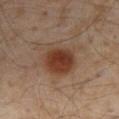{"biopsy_status": "not biopsied; imaged during a skin examination", "lesion_size": {"long_diameter_mm_approx": 5.0}, "site": "abdomen", "patient": {"sex": "male", "age_approx": 60}, "image": {"source": "total-body photography crop", "field_of_view_mm": 15}, "automated_metrics": {"area_mm2_approx": 16.0, "eccentricity": 0.45, "shape_asymmetry": 0.15, "cielab_L": 35, "cielab_a": 18, "cielab_b": 26, "vs_skin_darker_L": 10.0, "nevus_likeness_0_100": 100, "lesion_detection_confidence_0_100": 100}}